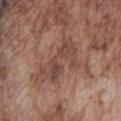notes: imaged on a skin check; not biopsied
location: the front of the torso
patient: male, in their mid- to late 70s
image: total-body-photography crop, ~15 mm field of view
lighting: white-light illumination
diameter: about 6 mm